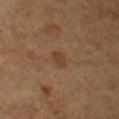Clinical impression: Recorded during total-body skin imaging; not selected for excision or biopsy.